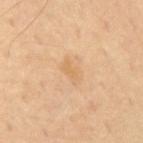{"biopsy_status": "not biopsied; imaged during a skin examination", "site": "mid back", "automated_metrics": {"nevus_likeness_0_100": 0, "lesion_detection_confidence_0_100": 100}, "lighting": "cross-polarized", "lesion_size": {"long_diameter_mm_approx": 3.0}, "image": {"source": "total-body photography crop", "field_of_view_mm": 15}, "patient": {"sex": "male", "age_approx": 70}}